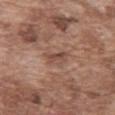Recorded during total-body skin imaging; not selected for excision or biopsy.
A male subject aged around 75.
Approximately 3 mm at its widest.
On the abdomen.
Automated image analysis of the tile measured two-axis asymmetry of about 0.45. It also reported a mean CIELAB color near L≈48 a*≈20 b*≈26, roughly 8 lightness units darker than nearby skin, and a normalized lesion–skin contrast near 6.
This is a white-light tile.
A roughly 15 mm field-of-view crop from a total-body skin photograph.